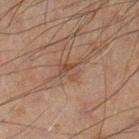biopsy_status: not biopsied; imaged during a skin examination
image:
  source: total-body photography crop
  field_of_view_mm: 15
patient:
  sex: male
  age_approx: 60
site: left leg
lesion_size:
  long_diameter_mm_approx: 3.0